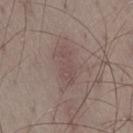Impression: Imaged during a routine full-body skin examination; the lesion was not biopsied and no histopathology is available. Image and clinical context: A roughly 15 mm field-of-view crop from a total-body skin photograph. On the right thigh. A male patient, aged 48 to 52. Approximately 4.5 mm at its widest.